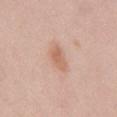The lesion was photographed on a routine skin check and not biopsied; there is no pathology result. Automated tile analysis of the lesion measured a footprint of about 5.5 mm², an eccentricity of roughly 0.85, and a shape-asymmetry score of about 0.25 (0 = symmetric). The analysis additionally found an average lesion color of about L≈63 a*≈21 b*≈30 (CIELAB) and a lesion–skin lightness drop of about 9. The analysis additionally found a classifier nevus-likeness of about 55/100 and a detector confidence of about 100 out of 100 that the crop contains a lesion. The patient is a female approximately 25 years of age. Longest diameter approximately 4 mm. The lesion is located on the abdomen. A close-up tile cropped from a whole-body skin photograph, about 15 mm across.